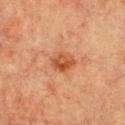workup — total-body-photography surveillance lesion; no biopsy
subject — female, aged 53–57
illumination — cross-polarized
site — the chest
acquisition — total-body-photography crop, ~15 mm field of view
automated lesion analysis — a footprint of about 5 mm² and two-axis asymmetry of about 0.15; a border-irregularity rating of about 1.5/10 and a color-variation rating of about 4.5/10